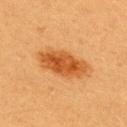Recorded during total-body skin imaging; not selected for excision or biopsy. The lesion-visualizer software estimated a lesion area of about 15 mm², a shape eccentricity near 0.9, and a shape-asymmetry score of about 0.2 (0 = symmetric). The software also gave a mean CIELAB color near L≈49 a*≈27 b*≈42, a lesion–skin lightness drop of about 12, and a normalized border contrast of about 8.5. The software also gave a border-irregularity rating of about 2.5/10, a color-variation rating of about 4.5/10, and a peripheral color-asymmetry measure near 1.5. The analysis additionally found a classifier nevus-likeness of about 100/100 and a lesion-detection confidence of about 100/100. A roughly 15 mm field-of-view crop from a total-body skin photograph. This is a cross-polarized tile. The patient is a female about 20 years old. The lesion is on the upper back.